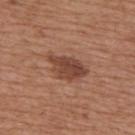Q: Is there a histopathology result?
A: imaged on a skin check; not biopsied
Q: How large is the lesion?
A: about 5.5 mm
Q: What are the patient's age and sex?
A: male, roughly 65 years of age
Q: What did automated image analysis measure?
A: a footprint of about 10 mm², an eccentricity of roughly 0.85, and two-axis asymmetry of about 0.3; a classifier nevus-likeness of about 75/100 and lesion-presence confidence of about 100/100
Q: Where on the body is the lesion?
A: the upper back
Q: What is the imaging modality?
A: ~15 mm tile from a whole-body skin photo